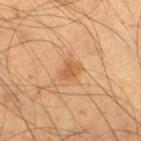Imaged during a routine full-body skin examination; the lesion was not biopsied and no histopathology is available.
A male patient aged 33–37.
From the right thigh.
A roughly 15 mm field-of-view crop from a total-body skin photograph.
This is a cross-polarized tile.